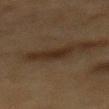Clinical impression: The lesion was photographed on a routine skin check and not biopsied; there is no pathology result. Image and clinical context: Cropped from a whole-body photographic skin survey; the tile spans about 15 mm. The lesion's longest dimension is about 3 mm. Imaged with cross-polarized lighting. The lesion is located on the mid back. The subject is a male aged around 85 or older.